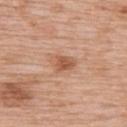notes = total-body-photography surveillance lesion; no biopsy
site = the back
lesion size = ≈3 mm
acquisition = ~15 mm tile from a whole-body skin photo
patient = female, in their 70s
lighting = white-light